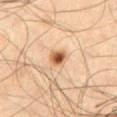Impression: No biopsy was performed on this lesion — it was imaged during a full skin examination and was not determined to be concerning. Background: A lesion tile, about 15 mm wide, cut from a 3D total-body photograph. A male subject roughly 55 years of age. From the chest.